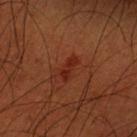<lesion>
  <biopsy_status>not biopsied; imaged during a skin examination</biopsy_status>
  <patient>
    <sex>male</sex>
    <age_approx>50</age_approx>
  </patient>
  <lighting>cross-polarized</lighting>
  <lesion_size>
    <long_diameter_mm_approx>3.0</long_diameter_mm_approx>
  </lesion_size>
  <image>
    <source>total-body photography crop</source>
    <field_of_view_mm>15</field_of_view_mm>
  </image>
  <site>left forearm</site>
</lesion>